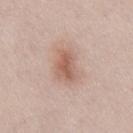Assessment: Captured during whole-body skin photography for melanoma surveillance; the lesion was not biopsied. Context: The patient is a male aged 48 to 52. A close-up tile cropped from a whole-body skin photograph, about 15 mm across. The lesion is on the abdomen.